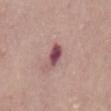{
  "biopsy_status": "not biopsied; imaged during a skin examination",
  "lesion_size": {
    "long_diameter_mm_approx": 3.5
  },
  "image": {
    "source": "total-body photography crop",
    "field_of_view_mm": 15
  },
  "patient": {
    "sex": "male",
    "age_approx": "85+"
  },
  "site": "mid back",
  "lighting": "white-light"
}